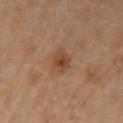Impression: Imaged during a routine full-body skin examination; the lesion was not biopsied and no histopathology is available. Background: A female subject aged 63 to 67. The tile uses cross-polarized illumination. The lesion is on the arm. The lesion's longest dimension is about 2.5 mm. Cropped from a total-body skin-imaging series; the visible field is about 15 mm. Automated image analysis of the tile measured a mean CIELAB color near L≈45 a*≈21 b*≈34, roughly 9 lightness units darker than nearby skin, and a normalized lesion–skin contrast near 7.5. The software also gave a border-irregularity index near 2/10, a color-variation rating of about 3.5/10, and peripheral color asymmetry of about 1. The analysis additionally found lesion-presence confidence of about 100/100.